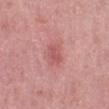Recorded during total-body skin imaging; not selected for excision or biopsy. On the left lower leg. A female subject aged 38 to 42. About 2.5 mm across. Automated image analysis of the tile measured border irregularity of about 3 on a 0–10 scale, a color-variation rating of about 1/10, and a peripheral color-asymmetry measure near 0.5. Cropped from a whole-body photographic skin survey; the tile spans about 15 mm.